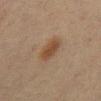Findings:
– biopsy status: imaged on a skin check; not biopsied
– site: the mid back
– subject: male, aged 68–72
– acquisition: total-body-photography crop, ~15 mm field of view
– illumination: cross-polarized illumination
– lesion diameter: ≈3.5 mm